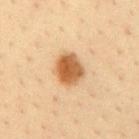Cropped from a whole-body photographic skin survey; the tile spans about 15 mm. The lesion is located on the back. The recorded lesion diameter is about 4 mm. A male patient, roughly 35 years of age.